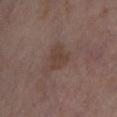biopsy status = total-body-photography surveillance lesion; no biopsy
patient = female, aged 53 to 57
diameter = about 3 mm
location = the left thigh
acquisition = ~15 mm crop, total-body skin-cancer survey
lighting = cross-polarized